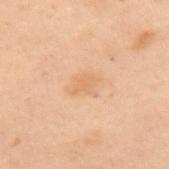notes — total-body-photography surveillance lesion; no biopsy | subject — female, aged approximately 55 | lighting — cross-polarized | acquisition — total-body-photography crop, ~15 mm field of view | anatomic site — the upper back.